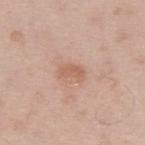Clinical impression:
The lesion was tiled from a total-body skin photograph and was not biopsied.
Image and clinical context:
This is a white-light tile. A male subject aged approximately 35. A 15 mm close-up tile from a total-body photography series done for melanoma screening. The lesion is on the left upper arm.